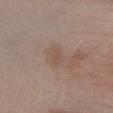Imaged during a routine full-body skin examination; the lesion was not biopsied and no histopathology is available.
Captured under white-light illumination.
Cropped from a total-body skin-imaging series; the visible field is about 15 mm.
The total-body-photography lesion software estimated a footprint of about 5 mm² and an outline eccentricity of about 0.6 (0 = round, 1 = elongated). The analysis additionally found a normalized border contrast of about 4.5.
On the leg.
The patient is a male in their 40s.